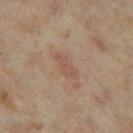Imaged during a routine full-body skin examination; the lesion was not biopsied and no histopathology is available. The lesion's longest dimension is about 3.5 mm. A female subject, approximately 35 years of age. Located on the left thigh. Automated image analysis of the tile measured border irregularity of about 3 on a 0–10 scale, a color-variation rating of about 2/10, and peripheral color asymmetry of about 0.5. Captured under cross-polarized illumination. Cropped from a whole-body photographic skin survey; the tile spans about 15 mm.